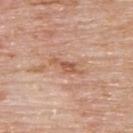No biopsy was performed on this lesion — it was imaged during a full skin examination and was not determined to be concerning. On the upper back. The lesion's longest dimension is about 4 mm. Automated tile analysis of the lesion measured internal color variation of about 1.5 on a 0–10 scale and peripheral color asymmetry of about 0.5. It also reported a classifier nevus-likeness of about 0/100 and lesion-presence confidence of about 100/100. A male subject aged approximately 75. This is a white-light tile. A 15 mm crop from a total-body photograph taken for skin-cancer surveillance.